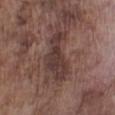About 6 mm across.
Cropped from a whole-body photographic skin survey; the tile spans about 15 mm.
Located on the leg.
The total-body-photography lesion software estimated a lesion color around L≈36 a*≈17 b*≈19 in CIELAB, about 9 CIELAB-L* units darker than the surrounding skin, and a normalized border contrast of about 8.
The tile uses white-light illumination.
A male patient, in their mid-70s.